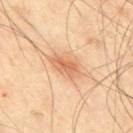biopsy_status: not biopsied; imaged during a skin examination
image:
  source: total-body photography crop
  field_of_view_mm: 15
site: back
patient:
  sex: male
  age_approx: 65